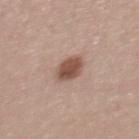Part of a total-body skin-imaging series; this lesion was reviewed on a skin check and was not flagged for biopsy. A close-up tile cropped from a whole-body skin photograph, about 15 mm across. Located on the back. Automated tile analysis of the lesion measured an area of roughly 6.5 mm² and an eccentricity of roughly 0.45. And it measured a lesion color around L≈51 a*≈20 b*≈26 in CIELAB, roughly 14 lightness units darker than nearby skin, and a lesion-to-skin contrast of about 9.5 (normalized; higher = more distinct). Approximately 3 mm at its widest. A female patient, aged approximately 25. Captured under white-light illumination.